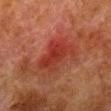Assessment:
Captured during whole-body skin photography for melanoma surveillance; the lesion was not biopsied.
Clinical summary:
Captured under cross-polarized illumination. About 5 mm across. From the right lower leg. This image is a 15 mm lesion crop taken from a total-body photograph. The patient is a male roughly 80 years of age. The total-body-photography lesion software estimated an average lesion color of about L≈29 a*≈28 b*≈26 (CIELAB), a lesion–skin lightness drop of about 7, and a lesion-to-skin contrast of about 7 (normalized; higher = more distinct). The analysis additionally found a border-irregularity index near 4/10 and a within-lesion color-variation index near 4/10.